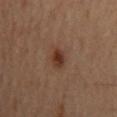Part of a total-body skin-imaging series; this lesion was reviewed on a skin check and was not flagged for biopsy.
From the mid back.
A lesion tile, about 15 mm wide, cut from a 3D total-body photograph.
A male subject, aged 58–62.
Imaged with cross-polarized lighting.
Longest diameter approximately 3 mm.